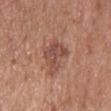Impression:
Captured during whole-body skin photography for melanoma surveillance; the lesion was not biopsied.
Clinical summary:
A roughly 15 mm field-of-view crop from a total-body skin photograph. This is a white-light tile. Located on the chest. The patient is a female in their 60s. Automated image analysis of the tile measured a lesion area of about 8.5 mm² and a symmetry-axis asymmetry near 0.4. It also reported an average lesion color of about L≈48 a*≈23 b*≈26 (CIELAB), a lesion–skin lightness drop of about 9, and a lesion-to-skin contrast of about 7 (normalized; higher = more distinct). The software also gave a within-lesion color-variation index near 4.5/10. The recorded lesion diameter is about 5 mm.